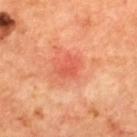notes: no biopsy performed (imaged during a skin exam)
automated metrics: a footprint of about 4.5 mm², a shape eccentricity near 0.7, and a shape-asymmetry score of about 0.3 (0 = symmetric)
anatomic site: the upper back
subject: aged 63 to 67
lighting: cross-polarized
lesion diameter: ≈3 mm
acquisition: ~15 mm crop, total-body skin-cancer survey